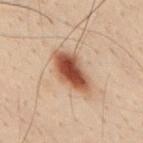Part of a total-body skin-imaging series; this lesion was reviewed on a skin check and was not flagged for biopsy.
A 15 mm close-up extracted from a 3D total-body photography capture.
From the mid back.
A male subject aged 28–32.